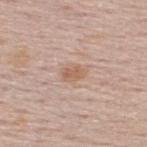Q: Was this lesion biopsied?
A: imaged on a skin check; not biopsied
Q: How large is the lesion?
A: about 2.5 mm
Q: What lighting was used for the tile?
A: white-light illumination
Q: What kind of image is this?
A: ~15 mm crop, total-body skin-cancer survey
Q: What are the patient's age and sex?
A: male, aged around 60
Q: What did automated image analysis measure?
A: an area of roughly 4 mm² and a shape-asymmetry score of about 0.2 (0 = symmetric); a mean CIELAB color near L≈60 a*≈19 b*≈30, a lesion–skin lightness drop of about 8, and a lesion-to-skin contrast of about 6.5 (normalized; higher = more distinct); a border-irregularity index near 2/10, a color-variation rating of about 1.5/10, and a peripheral color-asymmetry measure near 0.5
Q: Where on the body is the lesion?
A: the upper back